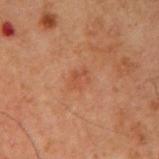<record>
  <biopsy_status>not biopsied; imaged during a skin examination</biopsy_status>
  <site>arm</site>
  <lesion_size>
    <long_diameter_mm_approx>2.5</long_diameter_mm_approx>
  </lesion_size>
  <patient>
    <sex>male</sex>
    <age_approx>60</age_approx>
  </patient>
  <lighting>cross-polarized</lighting>
  <image>
    <source>total-body photography crop</source>
    <field_of_view_mm>15</field_of_view_mm>
  </image>
</record>